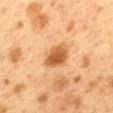Notes:
– workup · imaged on a skin check; not biopsied
– patient · female, approximately 40 years of age
– imaging modality · ~15 mm tile from a whole-body skin photo
– body site · the mid back
– illumination · cross-polarized illumination
– diameter · ~4 mm (longest diameter)